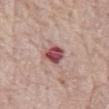Part of a total-body skin-imaging series; this lesion was reviewed on a skin check and was not flagged for biopsy.
The patient is a male roughly 80 years of age.
A 15 mm close-up extracted from a 3D total-body photography capture.
Captured under white-light illumination.
Longest diameter approximately 2.5 mm.
On the abdomen.